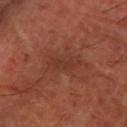{
  "biopsy_status": "not biopsied; imaged during a skin examination",
  "lesion_size": {
    "long_diameter_mm_approx": 4.0
  },
  "lighting": "cross-polarized",
  "image": {
    "source": "total-body photography crop",
    "field_of_view_mm": 15
  },
  "patient": {
    "age_approx": 65
  },
  "site": "arm"
}